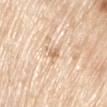No biopsy was performed on this lesion — it was imaged during a full skin examination and was not determined to be concerning. The lesion is located on the right upper arm. Automated tile analysis of the lesion measured a lesion area of about 3.5 mm² and a symmetry-axis asymmetry near 0.5. The software also gave internal color variation of about 3 on a 0–10 scale and a peripheral color-asymmetry measure near 1. The software also gave an automated nevus-likeness rating near 0 out of 100 and a detector confidence of about 90 out of 100 that the crop contains a lesion. The patient is a male aged around 80. Cropped from a whole-body photographic skin survey; the tile spans about 15 mm. The lesion's longest dimension is about 3 mm.